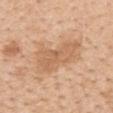Case summary:
- notes: imaged on a skin check; not biopsied
- imaging modality: total-body-photography crop, ~15 mm field of view
- location: the mid back
- patient: female, roughly 65 years of age